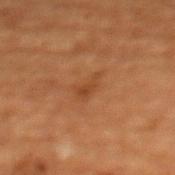– workup — imaged on a skin check; not biopsied
– location — the chest
– patient — female, aged approximately 80
– acquisition — ~15 mm tile from a whole-body skin photo
– lesion diameter — ≈2.5 mm